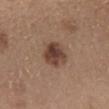<lesion>
<biopsy_status>not biopsied; imaged during a skin examination</biopsy_status>
<lesion_size>
  <long_diameter_mm_approx>3.5</long_diameter_mm_approx>
</lesion_size>
<lighting>white-light</lighting>
<image>
  <source>total-body photography crop</source>
  <field_of_view_mm>15</field_of_view_mm>
</image>
<patient>
  <sex>male</sex>
  <age_approx>55</age_approx>
</patient>
<site>back</site>
</lesion>